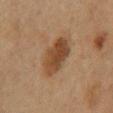- workup — no biopsy performed (imaged during a skin exam)
- anatomic site — the mid back
- automated metrics — a lesion area of about 13 mm², an eccentricity of roughly 0.8, and a shape-asymmetry score of about 0.2 (0 = symmetric); a mean CIELAB color near L≈40 a*≈17 b*≈30, about 10 CIELAB-L* units darker than the surrounding skin, and a lesion-to-skin contrast of about 9 (normalized; higher = more distinct); internal color variation of about 4 on a 0–10 scale and radial color variation of about 1.5
- subject — male, aged 58 to 62
- imaging modality — total-body-photography crop, ~15 mm field of view